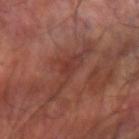| field | value |
|---|---|
| biopsy status | total-body-photography surveillance lesion; no biopsy |
| subject | male, approximately 60 years of age |
| automated lesion analysis | two-axis asymmetry of about 0.6; about 7 CIELAB-L* units darker than the surrounding skin and a normalized lesion–skin contrast near 6; a detector confidence of about 65 out of 100 that the crop contains a lesion |
| illumination | cross-polarized illumination |
| image | ~15 mm crop, total-body skin-cancer survey |
| diameter | ≈7 mm |
| body site | the arm |